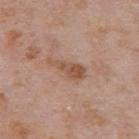The lesion is located on the mid back. Measured at roughly 4.5 mm in maximum diameter. The lesion-visualizer software estimated a lesion color around L≈52 a*≈20 b*≈30 in CIELAB, about 10 CIELAB-L* units darker than the surrounding skin, and a lesion-to-skin contrast of about 7.5 (normalized; higher = more distinct). It also reported a border-irregularity rating of about 5.5/10, a color-variation rating of about 3/10, and a peripheral color-asymmetry measure near 1. Imaged with white-light lighting. The subject is a male aged around 75. A roughly 15 mm field-of-view crop from a total-body skin photograph.